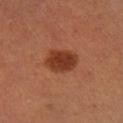Assessment:
Captured during whole-body skin photography for melanoma surveillance; the lesion was not biopsied.
Clinical summary:
A male subject, aged 48–52. The tile uses cross-polarized illumination. Approximately 4.5 mm at its widest. The lesion is on the right lower leg. The lesion-visualizer software estimated a lesion-detection confidence of about 100/100. A region of skin cropped from a whole-body photographic capture, roughly 15 mm wide.